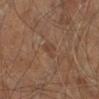imaging modality=~15 mm tile from a whole-body skin photo | site=the right leg | size=≈2.5 mm | subject=male, about 60 years old.